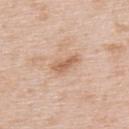| key | value |
|---|---|
| follow-up | catalogued during a skin exam; not biopsied |
| body site | the upper back |
| illumination | white-light illumination |
| subject | male, aged approximately 50 |
| automated metrics | a lesion area of about 3.5 mm², an outline eccentricity of about 0.9 (0 = round, 1 = elongated), and a shape-asymmetry score of about 0.35 (0 = symmetric); an average lesion color of about L≈61 a*≈20 b*≈33 (CIELAB), a lesion–skin lightness drop of about 11, and a normalized border contrast of about 7.5; a lesion-detection confidence of about 100/100 |
| imaging modality | 15 mm crop, total-body photography |